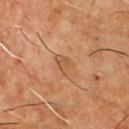No biopsy was performed on this lesion — it was imaged during a full skin examination and was not determined to be concerning. A close-up tile cropped from a whole-body skin photograph, about 15 mm across. A male patient roughly 60 years of age. About 3 mm across. Located on the chest. Captured under cross-polarized illumination. The lesion-visualizer software estimated an average lesion color of about L≈49 a*≈20 b*≈35 (CIELAB), about 6 CIELAB-L* units darker than the surrounding skin, and a lesion-to-skin contrast of about 5 (normalized; higher = more distinct). It also reported a border-irregularity index near 4/10, internal color variation of about 1 on a 0–10 scale, and radial color variation of about 0.5. The software also gave a nevus-likeness score of about 0/100.